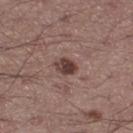Recorded during total-body skin imaging; not selected for excision or biopsy.
The lesion's longest dimension is about 2.5 mm.
The lesion-visualizer software estimated a footprint of about 4 mm², an eccentricity of roughly 0.65, and a symmetry-axis asymmetry near 0.2. It also reported an automated nevus-likeness rating near 90 out of 100 and a detector confidence of about 100 out of 100 that the crop contains a lesion.
The patient is a male roughly 55 years of age.
Located on the right thigh.
Cropped from a total-body skin-imaging series; the visible field is about 15 mm.
Imaged with white-light lighting.